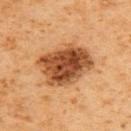Notes:
- follow-up · imaged on a skin check; not biopsied
- body site · the back
- TBP lesion metrics · a footprint of about 23 mm², an outline eccentricity of about 0.7 (0 = round, 1 = elongated), and a shape-asymmetry score of about 0.2 (0 = symmetric); a normalized border contrast of about 11.5
- diameter · ≈6 mm
- subject · male, aged approximately 60
- imaging modality · 15 mm crop, total-body photography
- illumination · cross-polarized illumination